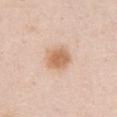notes=imaged on a skin check; not biopsied | patient=female, roughly 70 years of age | image=~15 mm tile from a whole-body skin photo | site=the chest.